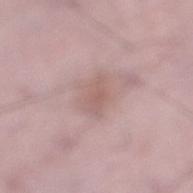The lesion was photographed on a routine skin check and not biopsied; there is no pathology result.
A close-up tile cropped from a whole-body skin photograph, about 15 mm across.
The lesion is on the leg.
The lesion's longest dimension is about 3.5 mm.
A male patient, aged 48–52.